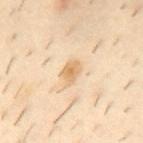Notes:
* notes — imaged on a skin check; not biopsied
* image source — ~15 mm crop, total-body skin-cancer survey
* image-analysis metrics — a color-variation rating of about 3/10 and radial color variation of about 1; an automated nevus-likeness rating near 15 out of 100 and lesion-presence confidence of about 100/100
* body site — the mid back
* patient — male, aged approximately 40